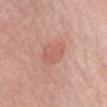notes — catalogued during a skin exam; not biopsied | lesion size — about 3.5 mm | subject — female, aged approximately 50 | illumination — white-light illumination | location — the chest | image — total-body-photography crop, ~15 mm field of view.